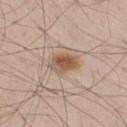  biopsy_status: not biopsied; imaged during a skin examination
  lighting: white-light
  site: left thigh
  image:
    source: total-body photography crop
    field_of_view_mm: 15
  patient:
    sex: male
    age_approx: 45
  automated_metrics:
    cielab_L: 55
    cielab_a: 18
    cielab_b: 29
    vs_skin_darker_L: 12.0
    vs_skin_contrast_norm: 8.5
    nevus_likeness_0_100: 95
    lesion_detection_confidence_0_100: 100
  lesion_size:
    long_diameter_mm_approx: 4.0